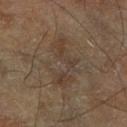{"lighting": "cross-polarized", "patient": {"sex": "male", "age_approx": 70}, "lesion_size": {"long_diameter_mm_approx": 6.5}, "automated_metrics": {"area_mm2_approx": 13.0, "eccentricity": 0.9, "shape_asymmetry": 0.45, "border_irregularity_0_10": 7.5, "color_variation_0_10": 3.5, "peripheral_color_asymmetry": 1.0, "nevus_likeness_0_100": 0, "lesion_detection_confidence_0_100": 65}, "site": "right lower leg", "image": {"source": "total-body photography crop", "field_of_view_mm": 15}}